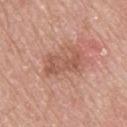Captured during whole-body skin photography for melanoma surveillance; the lesion was not biopsied. The lesion is located on the upper back. A region of skin cropped from a whole-body photographic capture, roughly 15 mm wide. Longest diameter approximately 5 mm. The subject is a male aged approximately 70.